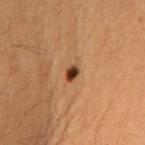Case summary:
* follow-up: imaged on a skin check; not biopsied
* diameter: about 2 mm
* anatomic site: the right upper arm
* patient: female, in their 40s
* image source: ~15 mm crop, total-body skin-cancer survey
* automated metrics: an area of roughly 2.5 mm², an eccentricity of roughly 0.7, and two-axis asymmetry of about 0.35; a nevus-likeness score of about 100/100 and a detector confidence of about 100 out of 100 that the crop contains a lesion
* illumination: cross-polarized illumination